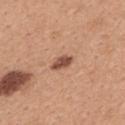A 15 mm close-up tile from a total-body photography series done for melanoma screening. The recorded lesion diameter is about 3 mm. This is a white-light tile. A female subject, about 30 years old. From the upper back.